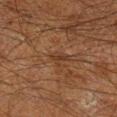Imaged during a routine full-body skin examination; the lesion was not biopsied and no histopathology is available.
The patient is a male about 60 years old.
A lesion tile, about 15 mm wide, cut from a 3D total-body photograph.
Automated tile analysis of the lesion measured a symmetry-axis asymmetry near 0.35.
The lesion is on the right lower leg.
The recorded lesion diameter is about 2.5 mm.